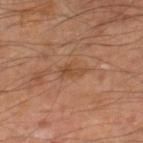No biopsy was performed on this lesion — it was imaged during a full skin examination and was not determined to be concerning.
A male patient, aged around 65.
The lesion is on the right lower leg.
Imaged with cross-polarized lighting.
A 15 mm crop from a total-body photograph taken for skin-cancer surveillance.
About 3 mm across.